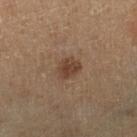follow-up = no biopsy performed (imaged during a skin exam); imaging modality = 15 mm crop, total-body photography; diameter = ≈2.5 mm; subject = about 60 years old; illumination = cross-polarized; body site = the right lower leg.